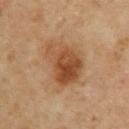workup — no biopsy performed (imaged during a skin exam) | anatomic site — the arm | imaging modality — 15 mm crop, total-body photography | subject — female, approximately 60 years of age.